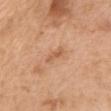Captured during whole-body skin photography for melanoma surveillance; the lesion was not biopsied.
The subject is a female roughly 55 years of age.
Automated tile analysis of the lesion measured a lesion area of about 3 mm², a shape eccentricity near 0.95, and two-axis asymmetry of about 0.35. It also reported a mean CIELAB color near L≈59 a*≈22 b*≈36 and about 8 CIELAB-L* units darker than the surrounding skin. The software also gave an automated nevus-likeness rating near 0 out of 100 and a lesion-detection confidence of about 100/100.
The tile uses white-light illumination.
This image is a 15 mm lesion crop taken from a total-body photograph.
The lesion is on the chest.
The recorded lesion diameter is about 3 mm.